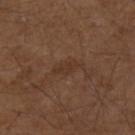| field | value |
|---|---|
| workup | catalogued during a skin exam; not biopsied |
| site | the arm |
| tile lighting | white-light illumination |
| image | ~15 mm crop, total-body skin-cancer survey |
| automated metrics | a lesion area of about 5 mm², an eccentricity of roughly 0.85, and two-axis asymmetry of about 0.25; a lesion color around L≈34 a*≈17 b*≈26 in CIELAB; border irregularity of about 3.5 on a 0–10 scale and a color-variation rating of about 1/10 |
| lesion diameter | about 3.5 mm |
| subject | male, aged 73–77 |